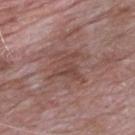Clinical impression:
Imaged during a routine full-body skin examination; the lesion was not biopsied and no histopathology is available.
Context:
The total-body-photography lesion software estimated a lesion color around L≈45 a*≈20 b*≈23 in CIELAB and a lesion–skin lightness drop of about 7. A male patient aged approximately 65. A 15 mm close-up extracted from a 3D total-body photography capture. About 4 mm across. The lesion is on the chest.